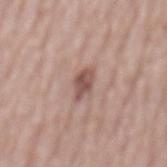workup=catalogued during a skin exam; not biopsied
subject=male, roughly 80 years of age
lighting=white-light
size=~3.5 mm (longest diameter)
acquisition=~15 mm crop, total-body skin-cancer survey
automated metrics=a mean CIELAB color near L≈53 a*≈19 b*≈23, a lesion–skin lightness drop of about 12, and a normalized lesion–skin contrast near 8; a border-irregularity rating of about 3/10 and a peripheral color-asymmetry measure near 1; a nevus-likeness score of about 15/100
anatomic site=the mid back